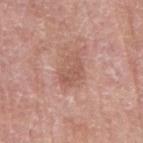Part of a total-body skin-imaging series; this lesion was reviewed on a skin check and was not flagged for biopsy.
Captured under white-light illumination.
A female patient aged approximately 60.
A close-up tile cropped from a whole-body skin photograph, about 15 mm across.
The lesion-visualizer software estimated a lesion color around L≈56 a*≈22 b*≈27 in CIELAB, a lesion–skin lightness drop of about 8, and a lesion-to-skin contrast of about 5.5 (normalized; higher = more distinct). And it measured border irregularity of about 5 on a 0–10 scale, internal color variation of about 2.5 on a 0–10 scale, and peripheral color asymmetry of about 1. The analysis additionally found lesion-presence confidence of about 100/100.
Measured at roughly 4 mm in maximum diameter.
The lesion is on the right upper arm.